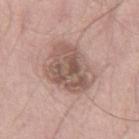Impression: Imaged during a routine full-body skin examination; the lesion was not biopsied and no histopathology is available. Context: The subject is a male in their 50s. A 15 mm close-up extracted from a 3D total-body photography capture. From the leg.